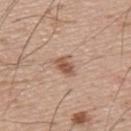Acquisition and patient details: A male patient, in their mid- to late 50s. Captured under white-light illumination. Cropped from a total-body skin-imaging series; the visible field is about 15 mm.